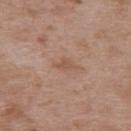site — the upper back | subject — male, approximately 50 years of age | image source — total-body-photography crop, ~15 mm field of view | lesion size — about 2.5 mm.